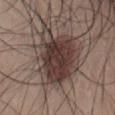  biopsy_status: not biopsied; imaged during a skin examination
  image:
    source: total-body photography crop
    field_of_view_mm: 15
  patient:
    sex: male
    age_approx: 35
  site: abdomen